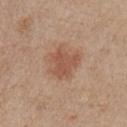Captured during whole-body skin photography for melanoma surveillance; the lesion was not biopsied. A male patient, about 75 years old. An algorithmic analysis of the crop reported a footprint of about 11 mm² and two-axis asymmetry of about 0.3. The analysis additionally found a lesion color around L≈53 a*≈21 b*≈30 in CIELAB, roughly 9 lightness units darker than nearby skin, and a normalized lesion–skin contrast near 6.5. It also reported an automated nevus-likeness rating near 75 out of 100 and a lesion-detection confidence of about 100/100. The tile uses white-light illumination. A 15 mm close-up tile from a total-body photography series done for melanoma screening. From the right upper arm.